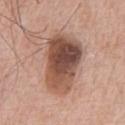workup = total-body-photography surveillance lesion; no biopsy